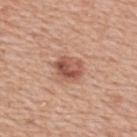Captured during whole-body skin photography for melanoma surveillance; the lesion was not biopsied. The lesion is on the upper back. A close-up tile cropped from a whole-body skin photograph, about 15 mm across. Imaged with white-light lighting. The patient is a female aged 48–52. The lesion-visualizer software estimated a mean CIELAB color near L≈54 a*≈24 b*≈29, about 12 CIELAB-L* units darker than the surrounding skin, and a normalized border contrast of about 8.5. The lesion's longest dimension is about 3.5 mm.